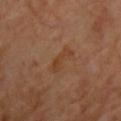Findings:
- follow-up · total-body-photography surveillance lesion; no biopsy